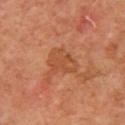No biopsy was performed on this lesion — it was imaged during a full skin examination and was not determined to be concerning. A female patient, roughly 40 years of age. A 15 mm crop from a total-body photograph taken for skin-cancer surveillance. Captured under cross-polarized illumination. The lesion is on the upper back. Measured at roughly 3.5 mm in maximum diameter.